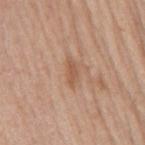biopsy_status: not biopsied; imaged during a skin examination
site: back
image:
  source: total-body photography crop
  field_of_view_mm: 15
automated_metrics:
  cielab_L: 56
  cielab_a: 20
  cielab_b: 32
  vs_skin_contrast_norm: 6.0
  border_irregularity_0_10: 3.5
  color_variation_0_10: 1.0
  peripheral_color_asymmetry: 0.5
  nevus_likeness_0_100: 0
lesion_size:
  long_diameter_mm_approx: 3.5
patient:
  sex: male
  age_approx: 65